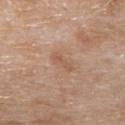{
  "biopsy_status": "not biopsied; imaged during a skin examination",
  "automated_metrics": {
    "nevus_likeness_0_100": 0,
    "lesion_detection_confidence_0_100": 100
  },
  "site": "chest",
  "image": {
    "source": "total-body photography crop",
    "field_of_view_mm": 15
  },
  "lighting": "white-light",
  "patient": {
    "sex": "male",
    "age_approx": 80
  },
  "lesion_size": {
    "long_diameter_mm_approx": 3.0
  }
}